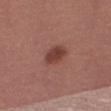Imaged during a routine full-body skin examination; the lesion was not biopsied and no histopathology is available.
The lesion is on the leg.
This is a white-light tile.
A female subject, approximately 55 years of age.
About 3 mm across.
A 15 mm crop from a total-body photograph taken for skin-cancer surveillance.